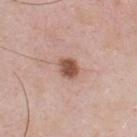Clinical impression: Captured during whole-body skin photography for melanoma surveillance; the lesion was not biopsied.